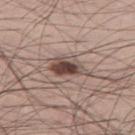| feature | finding |
|---|---|
| notes | no biopsy performed (imaged during a skin exam) |
| patient | male, aged around 30 |
| location | the left thigh |
| image | ~15 mm tile from a whole-body skin photo |